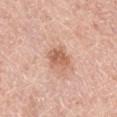No biopsy was performed on this lesion — it was imaged during a full skin examination and was not determined to be concerning. From the right lower leg. A 15 mm crop from a total-body photograph taken for skin-cancer surveillance. A female patient in their mid-60s.